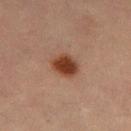The lesion is on the leg.
Imaged with cross-polarized lighting.
Cropped from a total-body skin-imaging series; the visible field is about 15 mm.
About 3 mm across.
A female patient, aged 68–72.